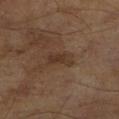Recorded during total-body skin imaging; not selected for excision or biopsy.
Captured under cross-polarized illumination.
The lesion's longest dimension is about 3.5 mm.
A roughly 15 mm field-of-view crop from a total-body skin photograph.
The patient is a male in their mid-60s.